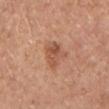acquisition=~15 mm crop, total-body skin-cancer survey | site=the chest | subject=male, about 55 years old.